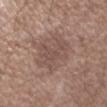• notes · catalogued during a skin exam; not biopsied
• tile lighting · white-light
• anatomic site · the left forearm
• acquisition · ~15 mm crop, total-body skin-cancer survey
• subject · male, aged 48 to 52
• diameter · about 5.5 mm